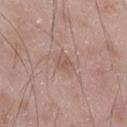Assessment:
This lesion was catalogued during total-body skin photography and was not selected for biopsy.
Image and clinical context:
From the leg. A close-up tile cropped from a whole-body skin photograph, about 15 mm across. Approximately 2.5 mm at its widest. Automated image analysis of the tile measured a lesion area of about 4 mm² and an eccentricity of roughly 0.65. The software also gave an average lesion color of about L≈55 a*≈19 b*≈24 (CIELAB) and a normalized lesion–skin contrast near 4.5. This is a white-light tile. A male patient approximately 50 years of age.